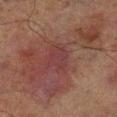{"biopsy_status": "not biopsied; imaged during a skin examination", "lesion_size": {"long_diameter_mm_approx": 12.0}, "lighting": "cross-polarized", "image": {"source": "total-body photography crop", "field_of_view_mm": 15}, "patient": {"sex": "male", "age_approx": 70}, "site": "right lower leg"}